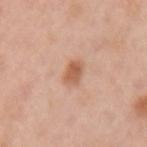follow-up — catalogued during a skin exam; not biopsied | subject — female, aged around 45 | location — the arm | image — 15 mm crop, total-body photography | illumination — white-light illumination.